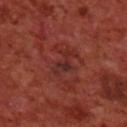{
  "image": {
    "source": "total-body photography crop",
    "field_of_view_mm": 15
  },
  "site": "upper back",
  "patient": {
    "sex": "male",
    "age_approx": 70
  }
}